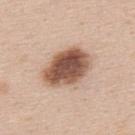Q: Is there a histopathology result?
A: no biopsy performed (imaged during a skin exam)
Q: Lesion location?
A: the upper back
Q: How was the tile lit?
A: white-light
Q: Who is the patient?
A: male, aged approximately 45
Q: What is the lesion's diameter?
A: ~6 mm (longest diameter)
Q: What is the imaging modality?
A: 15 mm crop, total-body photography